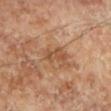Q: Was this lesion biopsied?
A: imaged on a skin check; not biopsied
Q: Who is the patient?
A: male, aged 68 to 72
Q: What kind of image is this?
A: ~15 mm tile from a whole-body skin photo
Q: Lesion location?
A: the left lower leg
Q: What lighting was used for the tile?
A: cross-polarized
Q: How large is the lesion?
A: about 3.5 mm